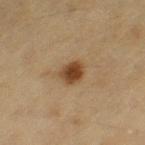Findings:
- notes — imaged on a skin check; not biopsied
- site — the left thigh
- size — ≈3 mm
- imaging modality — ~15 mm crop, total-body skin-cancer survey
- tile lighting — cross-polarized illumination
- automated lesion analysis — an automated nevus-likeness rating near 100 out of 100 and a detector confidence of about 100 out of 100 that the crop contains a lesion
- subject — female, in their mid- to late 50s